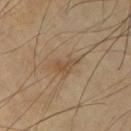workup: total-body-photography surveillance lesion; no biopsy | image: ~15 mm tile from a whole-body skin photo | illumination: cross-polarized illumination | lesion diameter: about 2.5 mm | patient: male, aged 63–67 | site: the right upper arm.